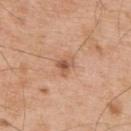Assessment:
No biopsy was performed on this lesion — it was imaged during a full skin examination and was not determined to be concerning.
Background:
On the upper back. A region of skin cropped from a whole-body photographic capture, roughly 15 mm wide. A male subject, aged approximately 55. Automated tile analysis of the lesion measured a mean CIELAB color near L≈57 a*≈22 b*≈33, a lesion–skin lightness drop of about 10, and a lesion-to-skin contrast of about 7 (normalized; higher = more distinct). Captured under white-light illumination. Longest diameter approximately 3 mm.